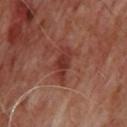notes: imaged on a skin check; not biopsied
tile lighting: cross-polarized
image source: ~15 mm tile from a whole-body skin photo
subject: male, aged around 65
site: the back
lesion size: ≈4.5 mm